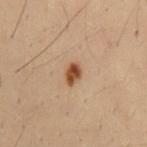notes = no biopsy performed (imaged during a skin exam); lesion size = ≈2.5 mm; acquisition = total-body-photography crop, ~15 mm field of view; patient = male, aged 28 to 32; body site = the mid back.